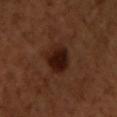Q: Was a biopsy performed?
A: no biopsy performed (imaged during a skin exam)
Q: How large is the lesion?
A: about 4 mm
Q: What did automated image analysis measure?
A: an area of roughly 9.5 mm² and a shape-asymmetry score of about 0.15 (0 = symmetric)
Q: Patient demographics?
A: male, aged approximately 50
Q: Where on the body is the lesion?
A: the chest
Q: What is the imaging modality?
A: ~15 mm crop, total-body skin-cancer survey